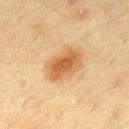The lesion was photographed on a routine skin check and not biopsied; there is no pathology result. From the back. A male subject, aged around 70. Cropped from a total-body skin-imaging series; the visible field is about 15 mm. An algorithmic analysis of the crop reported an area of roughly 11 mm² and a shape-asymmetry score of about 0.2 (0 = symmetric). And it measured a mean CIELAB color near L≈50 a*≈18 b*≈36 and a lesion–skin lightness drop of about 11. It also reported radial color variation of about 1.5. The lesion's longest dimension is about 4.5 mm.